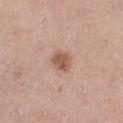  biopsy_status: not biopsied; imaged during a skin examination
  site: leg
  automated_metrics:
    border_irregularity_0_10: 2.0
    color_variation_0_10: 3.0
  image:
    source: total-body photography crop
    field_of_view_mm: 15
  patient:
    sex: female
    age_approx: 60
  lighting: white-light